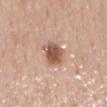Q: Was this lesion biopsied?
A: catalogued during a skin exam; not biopsied
Q: What is the imaging modality?
A: 15 mm crop, total-body photography
Q: What lighting was used for the tile?
A: white-light illumination
Q: What did automated image analysis measure?
A: an average lesion color of about L≈54 a*≈22 b*≈29 (CIELAB) and roughly 15 lightness units darker than nearby skin; a border-irregularity rating of about 2/10, a within-lesion color-variation index near 3.5/10, and a peripheral color-asymmetry measure near 1; a classifier nevus-likeness of about 95/100 and lesion-presence confidence of about 100/100
Q: Who is the patient?
A: female, aged 48–52
Q: Where on the body is the lesion?
A: the mid back